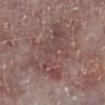automated lesion analysis = a mean CIELAB color near L≈46 a*≈18 b*≈19, roughly 7 lightness units darker than nearby skin, and a normalized border contrast of about 5.5; an automated nevus-likeness rating near 0 out of 100 and a lesion-detection confidence of about 85/100
patient = male, about 75 years old
anatomic site = the left lower leg
size = ~9 mm (longest diameter)
acquisition = 15 mm crop, total-body photography
tile lighting = white-light